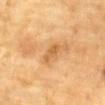Part of a total-body skin-imaging series; this lesion was reviewed on a skin check and was not flagged for biopsy.
Automated tile analysis of the lesion measured a footprint of about 3 mm² and an eccentricity of roughly 0.9. And it measured a mean CIELAB color near L≈52 a*≈20 b*≈40, a lesion–skin lightness drop of about 8, and a normalized border contrast of about 6.
A male subject, aged 83 or over.
Cropped from a whole-body photographic skin survey; the tile spans about 15 mm.
The lesion's longest dimension is about 3 mm.
Captured under cross-polarized illumination.
From the chest.